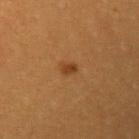Q: Is there a histopathology result?
A: catalogued during a skin exam; not biopsied
Q: Lesion size?
A: ≈2 mm
Q: How was this image acquired?
A: total-body-photography crop, ~15 mm field of view
Q: Lesion location?
A: the left upper arm
Q: Automated lesion metrics?
A: an area of roughly 2 mm², an eccentricity of roughly 0.8, and a symmetry-axis asymmetry near 0.25; an average lesion color of about L≈37 a*≈22 b*≈36 (CIELAB) and a normalized border contrast of about 7.5; internal color variation of about 0.5 on a 0–10 scale and radial color variation of about 0
Q: Patient demographics?
A: female, approximately 30 years of age
Q: Illumination type?
A: cross-polarized illumination